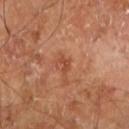Image and clinical context:
The subject is a male aged 58 to 62. Approximately 2.5 mm at its widest. An algorithmic analysis of the crop reported a border-irregularity index near 5/10, a within-lesion color-variation index near 0/10, and peripheral color asymmetry of about 0. A 15 mm close-up tile from a total-body photography series done for melanoma screening. Imaged with cross-polarized lighting. Located on the right leg.